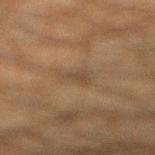Clinical impression:
The lesion was photographed on a routine skin check and not biopsied; there is no pathology result.
Clinical summary:
Measured at roughly 3.5 mm in maximum diameter. A male patient in their mid- to late 30s. An algorithmic analysis of the crop reported an area of roughly 5.5 mm², an outline eccentricity of about 0.7 (0 = round, 1 = elongated), and a shape-asymmetry score of about 0.35 (0 = symmetric). The software also gave a mean CIELAB color near L≈35 a*≈11 b*≈24, about 4 CIELAB-L* units darker than the surrounding skin, and a lesion-to-skin contrast of about 4.5 (normalized; higher = more distinct). The lesion is on the right lower leg. A 15 mm close-up tile from a total-body photography series done for melanoma screening. Imaged with cross-polarized lighting.